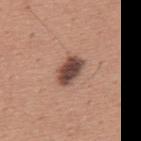No biopsy was performed on this lesion — it was imaged during a full skin examination and was not determined to be concerning.
About 4 mm across.
A close-up tile cropped from a whole-body skin photograph, about 15 mm across.
A male patient, aged around 45.
Imaged with white-light lighting.
On the mid back.